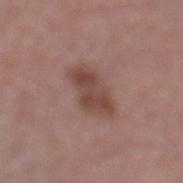Q: Is there a histopathology result?
A: total-body-photography surveillance lesion; no biopsy
Q: Who is the patient?
A: male, aged 73–77
Q: How was this image acquired?
A: 15 mm crop, total-body photography
Q: Lesion location?
A: the left lower leg